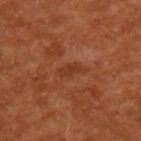notes = imaged on a skin check; not biopsied
patient = male, aged approximately 65
diameter = about 3 mm
imaging modality = 15 mm crop, total-body photography
anatomic site = the back
lighting = cross-polarized illumination